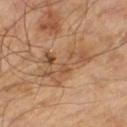Q: Is there a histopathology result?
A: no biopsy performed (imaged during a skin exam)
Q: What did automated image analysis measure?
A: a lesion area of about 10 mm², an outline eccentricity of about 0.85 (0 = round, 1 = elongated), and two-axis asymmetry of about 0.6; a lesion color around L≈50 a*≈19 b*≈32 in CIELAB and a normalized border contrast of about 6.5; a classifier nevus-likeness of about 0/100 and lesion-presence confidence of about 100/100
Q: How was this image acquired?
A: ~15 mm crop, total-body skin-cancer survey
Q: Patient demographics?
A: male, aged approximately 65
Q: How was the tile lit?
A: cross-polarized